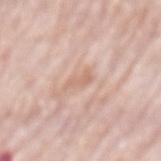Impression:
Imaged during a routine full-body skin examination; the lesion was not biopsied and no histopathology is available.
Context:
The patient is a male aged around 80. Cropped from a whole-body photographic skin survey; the tile spans about 15 mm. On the mid back. Measured at roughly 2.5 mm in maximum diameter.